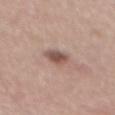Recorded during total-body skin imaging; not selected for excision or biopsy.
About 3.5 mm across.
A male patient, roughly 55 years of age.
The total-body-photography lesion software estimated an average lesion color of about L≈53 a*≈19 b*≈23 (CIELAB) and a normalized border contrast of about 8.5. The analysis additionally found a border-irregularity index near 2/10 and peripheral color asymmetry of about 1.5. The analysis additionally found an automated nevus-likeness rating near 85 out of 100 and a detector confidence of about 100 out of 100 that the crop contains a lesion.
Cropped from a whole-body photographic skin survey; the tile spans about 15 mm.
Captured under white-light illumination.
On the mid back.